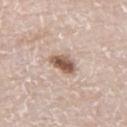Captured during whole-body skin photography for melanoma surveillance; the lesion was not biopsied.
An algorithmic analysis of the crop reported a lesion area of about 6 mm², an outline eccentricity of about 0.75 (0 = round, 1 = elongated), and a symmetry-axis asymmetry near 0.25. The analysis additionally found a lesion color around L≈56 a*≈17 b*≈27 in CIELAB, a lesion–skin lightness drop of about 16, and a normalized lesion–skin contrast near 10.5. The analysis additionally found a border-irregularity index near 2.5/10 and radial color variation of about 2. The software also gave a classifier nevus-likeness of about 95/100 and a detector confidence of about 100 out of 100 that the crop contains a lesion.
From the left thigh.
A male patient, approximately 80 years of age.
Cropped from a total-body skin-imaging series; the visible field is about 15 mm.
Measured at roughly 3 mm in maximum diameter.